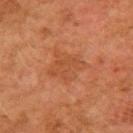| key | value |
|---|---|
| workup | imaged on a skin check; not biopsied |
| acquisition | ~15 mm tile from a whole-body skin photo |
| tile lighting | cross-polarized illumination |
| patient | female, aged 58–62 |
| site | the right upper arm |
| image-analysis metrics | an average lesion color of about L≈44 a*≈25 b*≈35 (CIELAB), a lesion–skin lightness drop of about 6, and a normalized lesion–skin contrast near 4.5; a classifier nevus-likeness of about 0/100 and a lesion-detection confidence of about 100/100 |
| size | ≈4.5 mm |